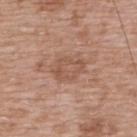Impression: No biopsy was performed on this lesion — it was imaged during a full skin examination and was not determined to be concerning. Acquisition and patient details: This is a white-light tile. On the upper back. A roughly 15 mm field-of-view crop from a total-body skin photograph. Automated image analysis of the tile measured a lesion color around L≈53 a*≈20 b*≈29 in CIELAB and about 7 CIELAB-L* units darker than the surrounding skin. The software also gave an automated nevus-likeness rating near 0 out of 100 and a detector confidence of about 100 out of 100 that the crop contains a lesion. A male subject, aged 48–52. The recorded lesion diameter is about 4 mm.